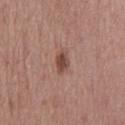No biopsy was performed on this lesion — it was imaged during a full skin examination and was not determined to be concerning.
An algorithmic analysis of the crop reported a nevus-likeness score of about 95/100.
A male subject, roughly 70 years of age.
On the mid back.
Longest diameter approximately 2.5 mm.
A roughly 15 mm field-of-view crop from a total-body skin photograph.